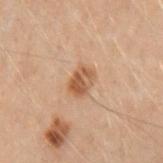Recorded during total-body skin imaging; not selected for excision or biopsy. About 3 mm across. A close-up tile cropped from a whole-body skin photograph, about 15 mm across. Automated tile analysis of the lesion measured an area of roughly 6 mm², an outline eccentricity of about 0.65 (0 = round, 1 = elongated), and a symmetry-axis asymmetry near 0.2. The analysis additionally found a mean CIELAB color near L≈44 a*≈17 b*≈29, roughly 9 lightness units darker than nearby skin, and a lesion-to-skin contrast of about 7.5 (normalized; higher = more distinct). It also reported a border-irregularity rating of about 2/10, a within-lesion color-variation index near 4/10, and peripheral color asymmetry of about 1.5. The tile uses cross-polarized illumination. The lesion is on the arm. A female subject, aged 28 to 32.